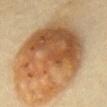No biopsy was performed on this lesion — it was imaged during a full skin examination and was not determined to be concerning. The recorded lesion diameter is about 16 mm. A roughly 15 mm field-of-view crop from a total-body skin photograph. A female subject aged 58–62. The lesion is on the front of the torso. An algorithmic analysis of the crop reported a border-irregularity index near 3.5/10, internal color variation of about 9 on a 0–10 scale, and peripheral color asymmetry of about 2.5.